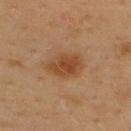Clinical impression:
Captured during whole-body skin photography for melanoma surveillance; the lesion was not biopsied.
Clinical summary:
The patient is a male aged approximately 40. The tile uses cross-polarized illumination. The lesion is located on the back. The lesion's longest dimension is about 4.5 mm. This image is a 15 mm lesion crop taken from a total-body photograph.